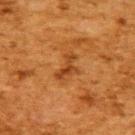Clinical impression: No biopsy was performed on this lesion — it was imaged during a full skin examination and was not determined to be concerning. Clinical summary: An algorithmic analysis of the crop reported an area of roughly 4.5 mm², a shape eccentricity near 0.85, and a shape-asymmetry score of about 0.55 (0 = symmetric). It also reported border irregularity of about 7 on a 0–10 scale, internal color variation of about 0 on a 0–10 scale, and peripheral color asymmetry of about 0. The lesion's longest dimension is about 3.5 mm. The tile uses cross-polarized illumination. A 15 mm close-up extracted from a 3D total-body photography capture. A female patient aged 48 to 52. Located on the upper back.